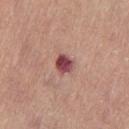<lesion>
  <biopsy_status>not biopsied; imaged during a skin examination</biopsy_status>
  <automated_metrics>
    <eccentricity>0.25</eccentricity>
    <shape_asymmetry>0.25</shape_asymmetry>
    <vs_skin_darker_L>16.0</vs_skin_darker_L>
    <color_variation_0_10>4.0</color_variation_0_10>
    <peripheral_color_asymmetry>1.0</peripheral_color_asymmetry>
    <nevus_likeness_0_100>25</nevus_likeness_0_100>
    <lesion_detection_confidence_0_100>100</lesion_detection_confidence_0_100>
  </automated_metrics>
  <patient>
    <sex>female</sex>
    <age_approx>65</age_approx>
  </patient>
  <lighting>white-light</lighting>
  <image>
    <source>total-body photography crop</source>
    <field_of_view_mm>15</field_of_view_mm>
  </image>
  <site>left thigh</site>
  <lesion_size>
    <long_diameter_mm_approx>2.5</long_diameter_mm_approx>
  </lesion_size>
</lesion>